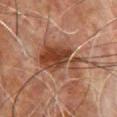notes = catalogued during a skin exam; not biopsied | image source = total-body-photography crop, ~15 mm field of view | body site = the chest | patient = male, aged approximately 65 | lesion diameter = ~7 mm (longest diameter) | illumination = cross-polarized.